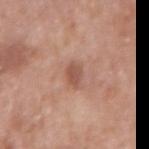workup = no biopsy performed (imaged during a skin exam) | location = the arm | size = about 2.5 mm | automated metrics = a mean CIELAB color near L≈53 a*≈22 b*≈28 and a normalized lesion–skin contrast near 7; a border-irregularity index near 2/10 and a color-variation rating of about 2.5/10; a nevus-likeness score of about 25/100 | patient = male, approximately 70 years of age | image = ~15 mm crop, total-body skin-cancer survey.